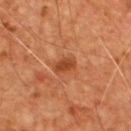Assessment: Imaged during a routine full-body skin examination; the lesion was not biopsied and no histopathology is available. Clinical summary: Approximately 2.5 mm at its widest. This is a cross-polarized tile. The subject is a male aged 53–57. A lesion tile, about 15 mm wide, cut from a 3D total-body photograph. On the chest. An algorithmic analysis of the crop reported an average lesion color of about L≈42 a*≈27 b*≈37 (CIELAB). It also reported peripheral color asymmetry of about 0.5.